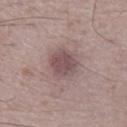notes — catalogued during a skin exam; not biopsied
patient — male, about 75 years old
image source — total-body-photography crop, ~15 mm field of view
site — the right lower leg